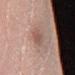Assessment:
No biopsy was performed on this lesion — it was imaged during a full skin examination and was not determined to be concerning.
Clinical summary:
A roughly 15 mm field-of-view crop from a total-body skin photograph. A female subject, aged around 45. The lesion's longest dimension is about 3 mm. On the right lower leg.